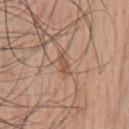<record>
<site>chest</site>
<automated_metrics>
  <area_mm2_approx>2.5</area_mm2_approx>
  <eccentricity>0.95</eccentricity>
  <shape_asymmetry>0.2</shape_asymmetry>
  <border_irregularity_0_10>2.5</border_irregularity_0_10>
  <color_variation_0_10>0.0</color_variation_0_10>
  <peripheral_color_asymmetry>0.0</peripheral_color_asymmetry>
</automated_metrics>
<image>
  <source>total-body photography crop</source>
  <field_of_view_mm>15</field_of_view_mm>
</image>
<patient>
  <sex>male</sex>
  <age_approx>55</age_approx>
</patient>
<lighting>white-light</lighting>
</record>